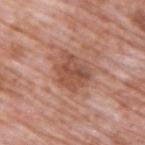This lesion was catalogued during total-body skin photography and was not selected for biopsy. The tile uses white-light illumination. A male subject, approximately 70 years of age. A close-up tile cropped from a whole-body skin photograph, about 15 mm across. Automated tile analysis of the lesion measured an area of roughly 11 mm², a shape eccentricity near 0.6, and two-axis asymmetry of about 0.25. And it measured a border-irregularity rating of about 3.5/10, a color-variation rating of about 4.5/10, and peripheral color asymmetry of about 1.5. Longest diameter approximately 4 mm. From the upper back.